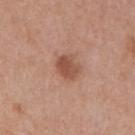Impression:
Captured during whole-body skin photography for melanoma surveillance; the lesion was not biopsied.